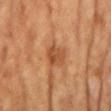Q: Was this lesion biopsied?
A: total-body-photography surveillance lesion; no biopsy
Q: What are the patient's age and sex?
A: female, roughly 65 years of age
Q: Lesion size?
A: ≈2.5 mm
Q: What is the anatomic site?
A: the chest
Q: Automated lesion metrics?
A: a footprint of about 5.5 mm², an outline eccentricity of about 0.3 (0 = round, 1 = elongated), and a symmetry-axis asymmetry near 0.25
Q: What is the imaging modality?
A: ~15 mm tile from a whole-body skin photo